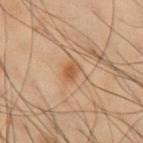Impression:
The lesion was photographed on a routine skin check and not biopsied; there is no pathology result.
Image and clinical context:
An algorithmic analysis of the crop reported an area of roughly 3.5 mm², a shape eccentricity near 0.75, and a symmetry-axis asymmetry near 0.25. And it measured border irregularity of about 2 on a 0–10 scale, a within-lesion color-variation index near 3/10, and a peripheral color-asymmetry measure near 1. The software also gave a nevus-likeness score of about 80/100 and a detector confidence of about 100 out of 100 that the crop contains a lesion. Longest diameter approximately 2.5 mm. On the front of the torso. Imaged with cross-polarized lighting. A lesion tile, about 15 mm wide, cut from a 3D total-body photograph. A male subject, aged 53–57.